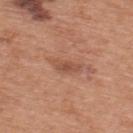The lesion is located on the upper back. Measured at roughly 3.5 mm in maximum diameter. A male subject aged 68–72. Captured under white-light illumination. A close-up tile cropped from a whole-body skin photograph, about 15 mm across.